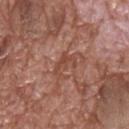- biopsy status — imaged on a skin check; not biopsied
- tile lighting — white-light
- site — the upper back
- automated metrics — an automated nevus-likeness rating near 0 out of 100
- acquisition — ~15 mm tile from a whole-body skin photo
- patient — male, aged 68 to 72